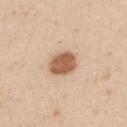A male patient, roughly 25 years of age. Captured under white-light illumination. The lesion's longest dimension is about 3.5 mm. Located on the upper back. A close-up tile cropped from a whole-body skin photograph, about 15 mm across.